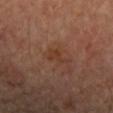Findings:
– notes: no biopsy performed (imaged during a skin exam)
– size: ≈3 mm
– subject: female, aged around 65
– acquisition: 15 mm crop, total-body photography
– site: the right forearm
– lighting: cross-polarized illumination